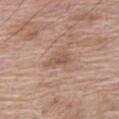Captured during whole-body skin photography for melanoma surveillance; the lesion was not biopsied. A region of skin cropped from a whole-body photographic capture, roughly 15 mm wide. From the leg. The lesion-visualizer software estimated a mean CIELAB color near L≈54 a*≈19 b*≈27, about 8 CIELAB-L* units darker than the surrounding skin, and a normalized border contrast of about 6. It also reported internal color variation of about 1 on a 0–10 scale and a peripheral color-asymmetry measure near 0.5. And it measured a nevus-likeness score of about 0/100 and a detector confidence of about 100 out of 100 that the crop contains a lesion. About 2.5 mm across. The tile uses white-light illumination. The patient is a female aged approximately 75.